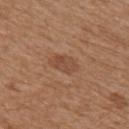Assessment: Part of a total-body skin-imaging series; this lesion was reviewed on a skin check and was not flagged for biopsy. Acquisition and patient details: A female patient, aged approximately 40. The total-body-photography lesion software estimated a lesion area of about 5.5 mm² and a shape-asymmetry score of about 0.25 (0 = symmetric). It also reported border irregularity of about 2.5 on a 0–10 scale and a color-variation rating of about 2.5/10. The analysis additionally found a nevus-likeness score of about 60/100 and lesion-presence confidence of about 100/100. The lesion is located on the right upper arm. Captured under white-light illumination. A 15 mm close-up tile from a total-body photography series done for melanoma screening.